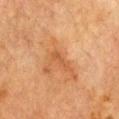<case>
  <site>chest</site>
  <patient>
    <sex>male</sex>
    <age_approx>85+</age_approx>
  </patient>
  <lighting>cross-polarized</lighting>
  <image>
    <source>total-body photography crop</source>
    <field_of_view_mm>15</field_of_view_mm>
  </image>
  <lesion_size>
    <long_diameter_mm_approx>3.0</long_diameter_mm_approx>
  </lesion_size>
</case>